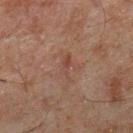This is a cross-polarized tile. The lesion-visualizer software estimated a footprint of about 3.5 mm², a shape eccentricity near 0.9, and a symmetry-axis asymmetry near 0.5. It also reported an average lesion color of about L≈36 a*≈17 b*≈22 (CIELAB), about 5 CIELAB-L* units darker than the surrounding skin, and a normalized lesion–skin contrast near 5. And it measured border irregularity of about 6 on a 0–10 scale, a color-variation rating of about 0.5/10, and peripheral color asymmetry of about 0. The lesion is on the leg. The lesion's longest dimension is about 3 mm. A 15 mm crop from a total-body photograph taken for skin-cancer surveillance. A male subject in their mid- to late 40s.